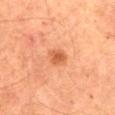Case summary:
• biopsy status · imaged on a skin check; not biopsied
• image source · ~15 mm crop, total-body skin-cancer survey
• patient · male, about 65 years old
• anatomic site · the abdomen